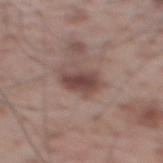workup: total-body-photography surveillance lesion; no biopsy | image-analysis metrics: a footprint of about 7.5 mm² and two-axis asymmetry of about 0.2; a border-irregularity rating of about 2.5/10, internal color variation of about 3 on a 0–10 scale, and peripheral color asymmetry of about 1 | diameter: ≈4 mm | image source: ~15 mm tile from a whole-body skin photo | subject: male, approximately 70 years of age | body site: the upper back | tile lighting: white-light illumination.